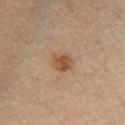Q: Is there a histopathology result?
A: no biopsy performed (imaged during a skin exam)
Q: How was this image acquired?
A: ~15 mm crop, total-body skin-cancer survey
Q: What are the patient's age and sex?
A: female, about 55 years old
Q: Where on the body is the lesion?
A: the right lower leg
Q: How was the tile lit?
A: cross-polarized illumination
Q: How large is the lesion?
A: ~3 mm (longest diameter)